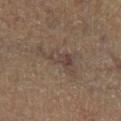follow-up: imaged on a skin check; not biopsied
image: 15 mm crop, total-body photography
site: the left lower leg
lesion diameter: ~5.5 mm (longest diameter)
illumination: cross-polarized illumination
patient: female, roughly 60 years of age